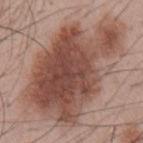Recorded during total-body skin imaging; not selected for excision or biopsy.
A male subject, in their mid-50s.
From the chest.
Approximately 13 mm at its widest.
A roughly 15 mm field-of-view crop from a total-body skin photograph.